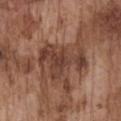{
  "biopsy_status": "not biopsied; imaged during a skin examination",
  "site": "chest",
  "patient": {
    "sex": "male",
    "age_approx": 75
  },
  "lesion_size": {
    "long_diameter_mm_approx": 6.0
  },
  "image": {
    "source": "total-body photography crop",
    "field_of_view_mm": 15
  }
}